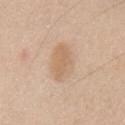biopsy_status: not biopsied; imaged during a skin examination
image:
  source: total-body photography crop
  field_of_view_mm: 15
site: front of the torso
patient:
  sex: male
  age_approx: 65